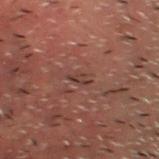A male patient, aged around 60. The lesion is on the head or neck. Longest diameter approximately 2.5 mm. A close-up tile cropped from a whole-body skin photograph, about 15 mm across.